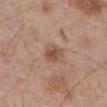Case summary:
- follow-up · catalogued during a skin exam; not biopsied
- location · the front of the torso
- acquisition · total-body-photography crop, ~15 mm field of view
- TBP lesion metrics · a shape eccentricity near 0.7; roughly 10 lightness units darker than nearby skin and a normalized border contrast of about 7.5; a classifier nevus-likeness of about 30/100 and lesion-presence confidence of about 100/100
- patient · male, approximately 70 years of age